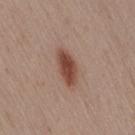Assessment:
Imaged during a routine full-body skin examination; the lesion was not biopsied and no histopathology is available.
Background:
The lesion is located on the lower back. The subject is a female roughly 30 years of age. An algorithmic analysis of the crop reported an area of roughly 8 mm², a shape eccentricity near 0.85, and a shape-asymmetry score of about 0.15 (0 = symmetric). A close-up tile cropped from a whole-body skin photograph, about 15 mm across. About 4.5 mm across.